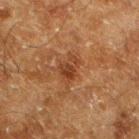Recorded during total-body skin imaging; not selected for excision or biopsy.
A male patient aged 58–62.
Imaged with cross-polarized lighting.
A lesion tile, about 15 mm wide, cut from a 3D total-body photograph.
Approximately 3 mm at its widest.
From the left lower leg.